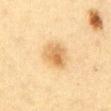Part of a total-body skin-imaging series; this lesion was reviewed on a skin check and was not flagged for biopsy. The subject is a female aged 53 to 57. Automated tile analysis of the lesion measured an average lesion color of about L≈58 a*≈16 b*≈38 (CIELAB), a lesion–skin lightness drop of about 10, and a normalized lesion–skin contrast near 7. And it measured a nevus-likeness score of about 95/100 and lesion-presence confidence of about 100/100. Longest diameter approximately 3.5 mm. This image is a 15 mm lesion crop taken from a total-body photograph. The lesion is located on the abdomen. The tile uses cross-polarized illumination.